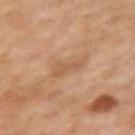Q: Was a biopsy performed?
A: total-body-photography surveillance lesion; no biopsy
Q: Lesion location?
A: the left upper arm
Q: How was this image acquired?
A: ~15 mm tile from a whole-body skin photo
Q: What lighting was used for the tile?
A: cross-polarized illumination
Q: Who is the patient?
A: female, in their 60s
Q: What is the lesion's diameter?
A: ≈3.5 mm